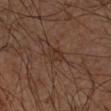About 3 mm across. The total-body-photography lesion software estimated a footprint of about 4.5 mm², an outline eccentricity of about 0.65 (0 = round, 1 = elongated), and a shape-asymmetry score of about 0.3 (0 = symmetric). And it measured an average lesion color of about L≈26 a*≈14 b*≈21 (CIELAB), about 5 CIELAB-L* units darker than the surrounding skin, and a normalized border contrast of about 5.5. Captured under cross-polarized illumination. A male patient, aged around 65. On the arm. A close-up tile cropped from a whole-body skin photograph, about 15 mm across.